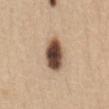Q: Was this lesion biopsied?
A: no biopsy performed (imaged during a skin exam)
Q: Who is the patient?
A: female, aged around 60
Q: Lesion location?
A: the front of the torso
Q: What is the imaging modality?
A: ~15 mm crop, total-body skin-cancer survey
Q: How large is the lesion?
A: about 4 mm
Q: What did automated image analysis measure?
A: an automated nevus-likeness rating near 100 out of 100 and a detector confidence of about 100 out of 100 that the crop contains a lesion